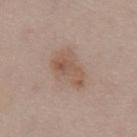Acquisition and patient details: The patient is a male aged around 55. Approximately 5 mm at its widest. On the front of the torso. This is a white-light tile. A close-up tile cropped from a whole-body skin photograph, about 15 mm across.